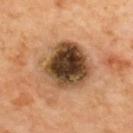The lesion was photographed on a routine skin check and not biopsied; there is no pathology result. A close-up tile cropped from a whole-body skin photograph, about 15 mm across. The lesion is located on the upper back. Approximately 6 mm at its widest. The lesion-visualizer software estimated an average lesion color of about L≈45 a*≈18 b*≈33 (CIELAB), roughly 21 lightness units darker than nearby skin, and a normalized lesion–skin contrast near 14.5. The software also gave a border-irregularity index near 2/10 and a within-lesion color-variation index near 10/10. And it measured a classifier nevus-likeness of about 0/100 and lesion-presence confidence of about 100/100. Captured under cross-polarized illumination.